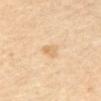The lesion was photographed on a routine skin check and not biopsied; there is no pathology result. A 15 mm close-up extracted from a 3D total-body photography capture. The lesion is on the abdomen. A female patient, in their mid-50s. The tile uses cross-polarized illumination. The recorded lesion diameter is about 2.5 mm. An algorithmic analysis of the crop reported a border-irregularity rating of about 2/10, a color-variation rating of about 2.5/10, and peripheral color asymmetry of about 1. It also reported a nevus-likeness score of about 0/100 and a detector confidence of about 100 out of 100 that the crop contains a lesion.